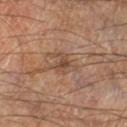Part of a total-body skin-imaging series; this lesion was reviewed on a skin check and was not flagged for biopsy.
Automated tile analysis of the lesion measured a lesion–skin lightness drop of about 8 and a normalized border contrast of about 6. And it measured a color-variation rating of about 3.5/10 and peripheral color asymmetry of about 1. The analysis additionally found an automated nevus-likeness rating near 0 out of 100 and a detector confidence of about 100 out of 100 that the crop contains a lesion.
The lesion is on the right lower leg.
Cropped from a total-body skin-imaging series; the visible field is about 15 mm.
The lesion's longest dimension is about 3.5 mm.
Imaged with cross-polarized lighting.
A male patient in their 70s.